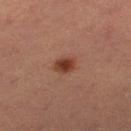Acquisition and patient details:
A lesion tile, about 15 mm wide, cut from a 3D total-body photograph. The tile uses cross-polarized illumination. The patient is a female in their mid- to late 50s. On the right thigh. Approximately 2.5 mm at its widest.